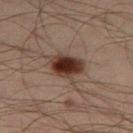biopsy status=no biopsy performed (imaged during a skin exam)
acquisition=~15 mm crop, total-body skin-cancer survey
location=the leg
size=≈4.5 mm
patient=male, in their mid- to late 30s
automated lesion analysis=border irregularity of about 2.5 on a 0–10 scale, a within-lesion color-variation index near 6.5/10, and a peripheral color-asymmetry measure near 2; a classifier nevus-likeness of about 100/100